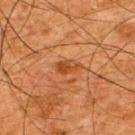Part of a total-body skin-imaging series; this lesion was reviewed on a skin check and was not flagged for biopsy. A male patient, aged 63–67. A close-up tile cropped from a whole-body skin photograph, about 15 mm across. Longest diameter approximately 3 mm. Automated image analysis of the tile measured an average lesion color of about L≈39 a*≈24 b*≈36 (CIELAB) and a lesion-to-skin contrast of about 7 (normalized; higher = more distinct). The analysis additionally found a border-irregularity rating of about 2/10 and a peripheral color-asymmetry measure near 1. It also reported a detector confidence of about 100 out of 100 that the crop contains a lesion. The lesion is located on the back. The tile uses cross-polarized illumination.